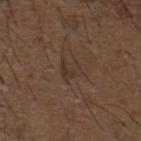notes: total-body-photography surveillance lesion; no biopsy
subject: male, aged 48–52
tile lighting: white-light illumination
site: the upper back
diameter: about 3 mm
image-analysis metrics: a lesion area of about 4.5 mm², an outline eccentricity of about 0.7 (0 = round, 1 = elongated), and a shape-asymmetry score of about 0.4 (0 = symmetric); an average lesion color of about L≈34 a*≈14 b*≈23 (CIELAB) and roughly 5 lightness units darker than nearby skin
image: 15 mm crop, total-body photography